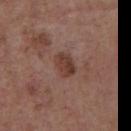| key | value |
|---|---|
| follow-up | no biopsy performed (imaged during a skin exam) |
| image | ~15 mm crop, total-body skin-cancer survey |
| illumination | white-light illumination |
| lesion size | about 3 mm |
| patient | male, aged around 55 |
| site | the chest |